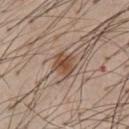Case summary:
* biopsy status: total-body-photography surveillance lesion; no biopsy